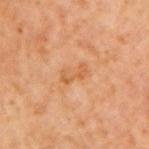body site: the right upper arm; acquisition: ~15 mm tile from a whole-body skin photo; subject: male, aged 58 to 62.